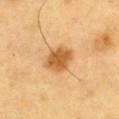Q: Is there a histopathology result?
A: catalogued during a skin exam; not biopsied
Q: What kind of image is this?
A: total-body-photography crop, ~15 mm field of view
Q: What is the anatomic site?
A: the chest
Q: What did automated image analysis measure?
A: an outline eccentricity of about 0.6 (0 = round, 1 = elongated); an average lesion color of about L≈59 a*≈23 b*≈45 (CIELAB), roughly 14 lightness units darker than nearby skin, and a normalized border contrast of about 9; a nevus-likeness score of about 100/100 and a detector confidence of about 100 out of 100 that the crop contains a lesion
Q: How large is the lesion?
A: ≈3.5 mm
Q: What lighting was used for the tile?
A: cross-polarized illumination
Q: What are the patient's age and sex?
A: male, about 60 years old